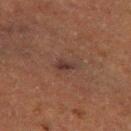The lesion was photographed on a routine skin check and not biopsied; there is no pathology result. The lesion is on the left thigh. A close-up tile cropped from a whole-body skin photograph, about 15 mm across. A male patient about 75 years old.